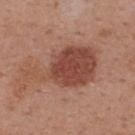{"biopsy_status": "not biopsied; imaged during a skin examination", "site": "upper back", "lighting": "white-light", "automated_metrics": {"area_mm2_approx": 27.0, "shape_asymmetry": 0.45, "cielab_L": 47, "cielab_a": 24, "cielab_b": 28, "vs_skin_darker_L": 11.0, "vs_skin_contrast_norm": 8.5, "nevus_likeness_0_100": 90, "lesion_detection_confidence_0_100": 100}, "patient": {"sex": "male", "age_approx": 55}, "image": {"source": "total-body photography crop", "field_of_view_mm": 15}}